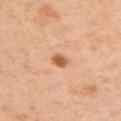The lesion was photographed on a routine skin check and not biopsied; there is no pathology result. The lesion is located on the left upper arm. Captured under cross-polarized illumination. Automated image analysis of the tile measured a mean CIELAB color near L≈59 a*≈25 b*≈38, about 13 CIELAB-L* units darker than the surrounding skin, and a normalized border contrast of about 8.5. A female subject, aged 38 to 42. Cropped from a total-body skin-imaging series; the visible field is about 15 mm. About 2 mm across.